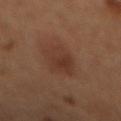Recorded during total-body skin imaging; not selected for excision or biopsy. A 15 mm close-up tile from a total-body photography series done for melanoma screening. Longest diameter approximately 5.5 mm. This is a cross-polarized tile. The subject is female. From the mid back.